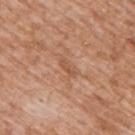imaging modality: 15 mm crop, total-body photography
body site: the upper back
patient: male, approximately 60 years of age
illumination: white-light illumination
lesion diameter: ≈2.5 mm